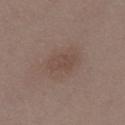This lesion was catalogued during total-body skin photography and was not selected for biopsy.
A 15 mm close-up tile from a total-body photography series done for melanoma screening.
On the chest.
Measured at roughly 4 mm in maximum diameter.
A female patient aged approximately 50.
The tile uses white-light illumination.
The lesion-visualizer software estimated an area of roughly 7 mm², an eccentricity of roughly 0.9, and a symmetry-axis asymmetry near 0.25.